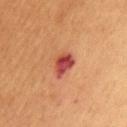Assessment: Part of a total-body skin-imaging series; this lesion was reviewed on a skin check and was not flagged for biopsy. Acquisition and patient details: Automated tile analysis of the lesion measured an area of roughly 6 mm², a shape eccentricity near 0.65, and two-axis asymmetry of about 0.25. It also reported a lesion color around L≈44 a*≈31 b*≈28 in CIELAB and a lesion–skin lightness drop of about 13. A male subject, aged 53 to 57. Cropped from a whole-body photographic skin survey; the tile spans about 15 mm. From the abdomen. This is a cross-polarized tile.